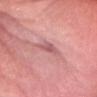No biopsy was performed on this lesion — it was imaged during a full skin examination and was not determined to be concerning. Captured under white-light illumination. A female patient, approximately 65 years of age. Automated image analysis of the tile measured an area of roughly 3.5 mm², an eccentricity of roughly 0.8, and a symmetry-axis asymmetry near 0.3. The software also gave a border-irregularity index near 2.5/10. The software also gave a classifier nevus-likeness of about 0/100. Cropped from a total-body skin-imaging series; the visible field is about 15 mm. The lesion is on the head or neck.